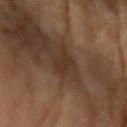Q: Was this lesion biopsied?
A: no biopsy performed (imaged during a skin exam)
Q: How large is the lesion?
A: about 3 mm
Q: How was this image acquired?
A: ~15 mm crop, total-body skin-cancer survey
Q: What are the patient's age and sex?
A: female, aged 58 to 62
Q: Where on the body is the lesion?
A: the left upper arm
Q: Illumination type?
A: cross-polarized illumination